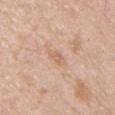biopsy_status: not biopsied; imaged during a skin examination
automated_metrics:
  color_variation_0_10: 1.0
  peripheral_color_asymmetry: 0.5
  nevus_likeness_0_100: 0
  lesion_detection_confidence_0_100: 100
patient:
  sex: male
  age_approx: 50
lighting: white-light
site: chest
image:
  source: total-body photography crop
  field_of_view_mm: 15
lesion_size:
  long_diameter_mm_approx: 3.0